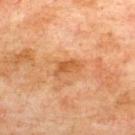Impression: No biopsy was performed on this lesion — it was imaged during a full skin examination and was not determined to be concerning. Context: Cropped from a total-body skin-imaging series; the visible field is about 15 mm. From the upper back. Imaged with cross-polarized lighting. Automated image analysis of the tile measured an eccentricity of roughly 0.85 and a symmetry-axis asymmetry near 0.4. It also reported radial color variation of about 1. And it measured an automated nevus-likeness rating near 0 out of 100. About 3.5 mm across. The subject is a male in their mid- to late 70s.